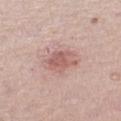Findings:
• follow-up — no biopsy performed (imaged during a skin exam)
• patient — female, roughly 40 years of age
• lesion diameter — about 3.5 mm
• body site — the left thigh
• image source — ~15 mm tile from a whole-body skin photo
• tile lighting — white-light